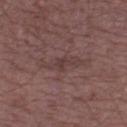Findings:
- follow-up: imaged on a skin check; not biopsied
- lighting: white-light
- imaging modality: 15 mm crop, total-body photography
- diameter: about 5 mm
- patient: male, roughly 50 years of age
- anatomic site: the leg